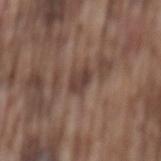biopsy_status: not biopsied; imaged during a skin examination
patient:
  sex: male
  age_approx: 75
image:
  source: total-body photography crop
  field_of_view_mm: 15
lesion_size:
  long_diameter_mm_approx: 2.5
site: mid back
automated_metrics:
  eccentricity: 0.55
  shape_asymmetry: 0.25
  cielab_L: 40
  cielab_a: 16
  cielab_b: 22
  vs_skin_contrast_norm: 8.0
  border_irregularity_0_10: 2.5
  peripheral_color_asymmetry: 1.0
  nevus_likeness_0_100: 0
  lesion_detection_confidence_0_100: 90
lighting: white-light